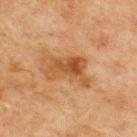Case summary:
* biopsy status — total-body-photography surveillance lesion; no biopsy
* anatomic site — the upper back
* imaging modality — ~15 mm crop, total-body skin-cancer survey
* illumination — cross-polarized
* patient — male, about 70 years old